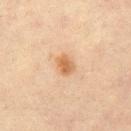A female subject, in their mid- to late 40s. A region of skin cropped from a whole-body photographic capture, roughly 15 mm wide. The tile uses cross-polarized illumination. The lesion is located on the left thigh. About 2.5 mm across. Automated tile analysis of the lesion measured a lesion area of about 4 mm² and an outline eccentricity of about 0.65 (0 = round, 1 = elongated). And it measured a border-irregularity rating of about 2/10, a color-variation rating of about 2.5/10, and radial color variation of about 1. And it measured a lesion-detection confidence of about 100/100.